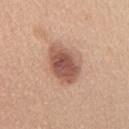{"biopsy_status": "not biopsied; imaged during a skin examination", "site": "upper back", "lesion_size": {"long_diameter_mm_approx": 5.5}, "automated_metrics": {"cielab_L": 55, "cielab_a": 22, "cielab_b": 28, "vs_skin_contrast_norm": 9.5, "border_irregularity_0_10": 2.0}, "lighting": "white-light", "image": {"source": "total-body photography crop", "field_of_view_mm": 15}, "patient": {"sex": "female", "age_approx": 40}}